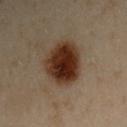| feature | finding |
|---|---|
| biopsy status | no biopsy performed (imaged during a skin exam) |
| lesion size | ~5.5 mm (longest diameter) |
| image | total-body-photography crop, ~15 mm field of view |
| location | the left arm |
| automated lesion analysis | a footprint of about 18 mm² and a shape-asymmetry score of about 0.15 (0 = symmetric); an average lesion color of about L≈24 a*≈16 b*≈23 (CIELAB), roughly 15 lightness units darker than nearby skin, and a normalized lesion–skin contrast near 15.5; an automated nevus-likeness rating near 100 out of 100 and lesion-presence confidence of about 100/100 |
| lighting | cross-polarized |
| patient | male, aged approximately 50 |